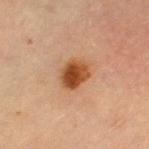subject: female, aged 68–72
image-analysis metrics: a mean CIELAB color near L≈42 a*≈23 b*≈34 and roughly 14 lightness units darker than nearby skin
illumination: cross-polarized illumination
lesion diameter: ~3.5 mm (longest diameter)
site: the left thigh
imaging modality: ~15 mm crop, total-body skin-cancer survey